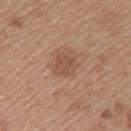follow-up = imaged on a skin check; not biopsied | diameter = ~2.5 mm (longest diameter) | subject = female, aged approximately 40 | location = the arm | image-analysis metrics = a lesion color around L≈51 a*≈20 b*≈28 in CIELAB and about 8 CIELAB-L* units darker than the surrounding skin; an automated nevus-likeness rating near 35 out of 100 and a detector confidence of about 100 out of 100 that the crop contains a lesion | image = 15 mm crop, total-body photography.